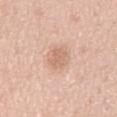Q: Was this lesion biopsied?
A: imaged on a skin check; not biopsied
Q: Patient demographics?
A: male, approximately 55 years of age
Q: What kind of image is this?
A: ~15 mm crop, total-body skin-cancer survey
Q: Lesion location?
A: the abdomen
Q: Illumination type?
A: white-light
Q: What is the lesion's diameter?
A: ~3 mm (longest diameter)